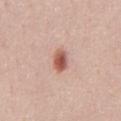Part of a total-body skin-imaging series; this lesion was reviewed on a skin check and was not flagged for biopsy.
Located on the front of the torso.
A region of skin cropped from a whole-body photographic capture, roughly 15 mm wide.
The patient is a male aged approximately 50.
Approximately 3 mm at its widest.
This is a white-light tile.
The lesion-visualizer software estimated a footprint of about 5 mm². And it measured border irregularity of about 2 on a 0–10 scale, a within-lesion color-variation index near 5.5/10, and peripheral color asymmetry of about 1.5. The software also gave a classifier nevus-likeness of about 95/100 and a lesion-detection confidence of about 100/100.